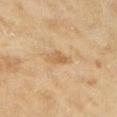Recorded during total-body skin imaging; not selected for excision or biopsy. On the leg. A lesion tile, about 15 mm wide, cut from a 3D total-body photograph. The recorded lesion diameter is about 2.5 mm. The total-body-photography lesion software estimated a mean CIELAB color near L≈55 a*≈16 b*≈36, about 8 CIELAB-L* units darker than the surrounding skin, and a lesion-to-skin contrast of about 6 (normalized; higher = more distinct). Captured under cross-polarized illumination. The patient is a female about 60 years old.